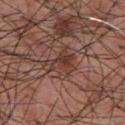Recorded during total-body skin imaging; not selected for excision or biopsy. A 15 mm crop from a total-body photograph taken for skin-cancer surveillance. On the front of the torso. An algorithmic analysis of the crop reported a lesion area of about 6.5 mm², a shape eccentricity near 0.8, and two-axis asymmetry of about 0.55. It also reported a lesion color around L≈38 a*≈20 b*≈24 in CIELAB, about 8 CIELAB-L* units darker than the surrounding skin, and a normalized lesion–skin contrast near 7. Approximately 4 mm at its widest. A male patient about 55 years old.